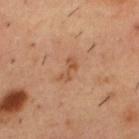Findings:
– subject: male, aged approximately 55
– site: the upper back
– tile lighting: cross-polarized
– acquisition: 15 mm crop, total-body photography